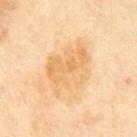Q: Is there a histopathology result?
A: no biopsy performed (imaged during a skin exam)
Q: What are the patient's age and sex?
A: male, aged around 70
Q: How was the tile lit?
A: cross-polarized illumination
Q: Automated lesion metrics?
A: an automated nevus-likeness rating near 0 out of 100 and a detector confidence of about 100 out of 100 that the crop contains a lesion
Q: How was this image acquired?
A: 15 mm crop, total-body photography
Q: Lesion location?
A: the front of the torso
Q: Lesion size?
A: ~7 mm (longest diameter)